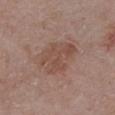The lesion was tiled from a total-body skin photograph and was not biopsied. The recorded lesion diameter is about 5 mm. An algorithmic analysis of the crop reported an average lesion color of about L≈48 a*≈19 b*≈25 (CIELAB). And it measured border irregularity of about 3.5 on a 0–10 scale, internal color variation of about 3 on a 0–10 scale, and a peripheral color-asymmetry measure near 1. Captured under white-light illumination. The subject is a female aged 48 to 52. On the chest. A lesion tile, about 15 mm wide, cut from a 3D total-body photograph.